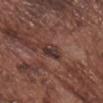– biopsy status: catalogued during a skin exam; not biopsied
– subject: male, aged 73–77
– imaging modality: ~15 mm tile from a whole-body skin photo
– body site: the head or neck
– size: ~3 mm (longest diameter)
– image-analysis metrics: a border-irregularity rating of about 3/10, a color-variation rating of about 3/10, and a peripheral color-asymmetry measure near 1